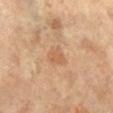Q: Was this lesion biopsied?
A: catalogued during a skin exam; not biopsied
Q: Illumination type?
A: cross-polarized illumination
Q: What is the lesion's diameter?
A: about 3 mm
Q: Where on the body is the lesion?
A: the left leg
Q: What did automated image analysis measure?
A: an area of roughly 5 mm², an eccentricity of roughly 0.65, and a symmetry-axis asymmetry near 0.35; an average lesion color of about L≈58 a*≈21 b*≈35 (CIELAB), about 7 CIELAB-L* units darker than the surrounding skin, and a normalized lesion–skin contrast near 5; a nevus-likeness score of about 0/100 and a lesion-detection confidence of about 100/100
Q: What are the patient's age and sex?
A: female, aged 63–67
Q: What is the imaging modality?
A: ~15 mm tile from a whole-body skin photo